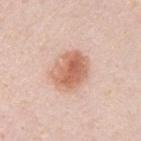follow-up: no biopsy performed (imaged during a skin exam) | size: about 4.5 mm | location: the upper back | tile lighting: white-light | automated metrics: a lesion area of about 13 mm², a shape eccentricity near 0.65, and a shape-asymmetry score of about 0.15 (0 = symmetric); a mean CIELAB color near L≈64 a*≈23 b*≈32, roughly 12 lightness units darker than nearby skin, and a normalized lesion–skin contrast near 8.5; a classifier nevus-likeness of about 95/100 and lesion-presence confidence of about 100/100 | image: total-body-photography crop, ~15 mm field of view | patient: male, approximately 35 years of age.